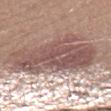Background:
This is a white-light tile. A 15 mm crop from a total-body photograph taken for skin-cancer surveillance. A male subject, aged approximately 30. From the left lower leg. The lesion-visualizer software estimated a lesion–skin lightness drop of about 14 and a normalized border contrast of about 9.5. The software also gave a lesion-detection confidence of about 30/100. Measured at roughly 10 mm in maximum diameter.